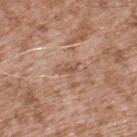| field | value |
|---|---|
| notes | no biopsy performed (imaged during a skin exam) |